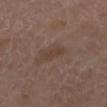Imaged during a routine full-body skin examination; the lesion was not biopsied and no histopathology is available.
This is a white-light tile.
On the right lower leg.
Measured at roughly 3 mm in maximum diameter.
A male subject, aged around 75.
A lesion tile, about 15 mm wide, cut from a 3D total-body photograph.